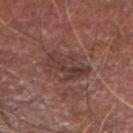The lesion was tiled from a total-body skin photograph and was not biopsied. The tile uses white-light illumination. Longest diameter approximately 4.5 mm. A male subject in their mid- to late 70s. Located on the arm. Cropped from a total-body skin-imaging series; the visible field is about 15 mm.